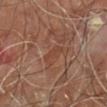Notes:
• biopsy status: no biopsy performed (imaged during a skin exam)
• patient: male, roughly 60 years of age
• image source: total-body-photography crop, ~15 mm field of view
• body site: the right leg
• lesion diameter: ~2.5 mm (longest diameter)